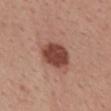biopsy status: catalogued during a skin exam; not biopsied | image source: 15 mm crop, total-body photography | diameter: ≈4 mm | location: the mid back | image-analysis metrics: a mean CIELAB color near L≈44 a*≈25 b*≈27 and a normalized lesion–skin contrast near 11 | patient: female, approximately 55 years of age.